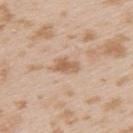Imaged during a routine full-body skin examination; the lesion was not biopsied and no histopathology is available.
A female subject, about 25 years old.
Measured at roughly 3 mm in maximum diameter.
On the left upper arm.
Imaged with white-light lighting.
A roughly 15 mm field-of-view crop from a total-body skin photograph.